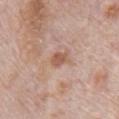Q: Is there a histopathology result?
A: imaged on a skin check; not biopsied
Q: What did automated image analysis measure?
A: a footprint of about 4 mm², a shape eccentricity near 0.7, and two-axis asymmetry of about 0.3; a lesion–skin lightness drop of about 9 and a normalized lesion–skin contrast near 7; a border-irregularity rating of about 2.5/10, internal color variation of about 1.5 on a 0–10 scale, and peripheral color asymmetry of about 0.5; a nevus-likeness score of about 0/100 and a lesion-detection confidence of about 100/100
Q: What is the imaging modality?
A: 15 mm crop, total-body photography
Q: Who is the patient?
A: male, about 70 years old
Q: Illumination type?
A: white-light illumination
Q: What is the anatomic site?
A: the abdomen
Q: How large is the lesion?
A: ~2.5 mm (longest diameter)